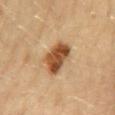biopsy status=catalogued during a skin exam; not biopsied
image=~15 mm tile from a whole-body skin photo
TBP lesion metrics=a normalized border contrast of about 11.5
illumination=cross-polarized
anatomic site=the mid back
lesion size=about 4.5 mm
patient=female, aged approximately 70